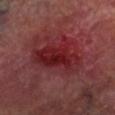Recorded during total-body skin imaging; not selected for excision or biopsy.